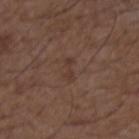Q: Was a biopsy performed?
A: total-body-photography surveillance lesion; no biopsy
Q: Patient demographics?
A: male, in their 50s
Q: Where on the body is the lesion?
A: the chest
Q: What kind of image is this?
A: total-body-photography crop, ~15 mm field of view